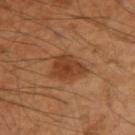Clinical impression: No biopsy was performed on this lesion — it was imaged during a full skin examination and was not determined to be concerning. Image and clinical context: The total-body-photography lesion software estimated a lesion color around L≈40 a*≈23 b*≈34 in CIELAB, about 10 CIELAB-L* units darker than the surrounding skin, and a normalized border contrast of about 8. The software also gave a border-irregularity index near 3/10, internal color variation of about 3 on a 0–10 scale, and a peripheral color-asymmetry measure near 1. The analysis additionally found a classifier nevus-likeness of about 65/100 and lesion-presence confidence of about 100/100. About 4 mm across. A male subject, aged 48 to 52. From the left arm. Imaged with cross-polarized lighting. Cropped from a total-body skin-imaging series; the visible field is about 15 mm.